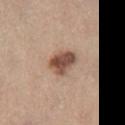The lesion was tiled from a total-body skin photograph and was not biopsied.
Captured under white-light illumination.
On the leg.
Measured at roughly 3.5 mm in maximum diameter.
The subject is a female in their mid- to late 60s.
This image is a 15 mm lesion crop taken from a total-body photograph.